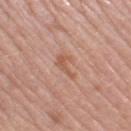Clinical impression: Captured during whole-body skin photography for melanoma surveillance; the lesion was not biopsied. Context: From the leg. A female patient, aged approximately 70. A 15 mm crop from a total-body photograph taken for skin-cancer surveillance.